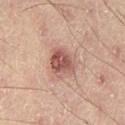Notes:
• workup · imaged on a skin check; not biopsied
• size · ≈4 mm
• body site · the left thigh
• lighting · cross-polarized
• subject · male, aged 48–52
• image source · ~15 mm crop, total-body skin-cancer survey